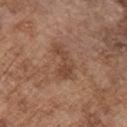About 5 mm across. Automated tile analysis of the lesion measured a shape eccentricity near 0.9. The software also gave a normalized lesion–skin contrast near 6.5. The software also gave a border-irregularity index near 6/10, internal color variation of about 2 on a 0–10 scale, and a peripheral color-asymmetry measure near 0.5. A 15 mm close-up extracted from a 3D total-body photography capture. A male subject aged 73 to 77. The tile uses white-light illumination. Located on the chest.